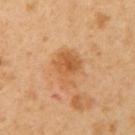Clinical summary: Cropped from a total-body skin-imaging series; the visible field is about 15 mm. Imaged with cross-polarized lighting. Approximately 4.5 mm at its widest. A female subject aged around 40. Automated image analysis of the tile measured a footprint of about 10 mm², a shape eccentricity near 0.75, and a symmetry-axis asymmetry near 0.3. And it measured border irregularity of about 3.5 on a 0–10 scale, a within-lesion color-variation index near 5/10, and radial color variation of about 1.5. It also reported a nevus-likeness score of about 20/100 and a detector confidence of about 100 out of 100 that the crop contains a lesion. The lesion is located on the left upper arm.